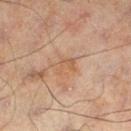No biopsy was performed on this lesion — it was imaged during a full skin examination and was not determined to be concerning. Imaged with cross-polarized lighting. Approximately 3 mm at its widest. A male subject, aged 43–47. A 15 mm close-up extracted from a 3D total-body photography capture. On the left lower leg.